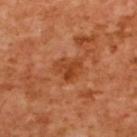Captured during whole-body skin photography for melanoma surveillance; the lesion was not biopsied. From the upper back. An algorithmic analysis of the crop reported a lesion area of about 7 mm², an outline eccentricity of about 0.65 (0 = round, 1 = elongated), and a shape-asymmetry score of about 0.35 (0 = symmetric). The software also gave border irregularity of about 3.5 on a 0–10 scale, a within-lesion color-variation index near 4.5/10, and peripheral color asymmetry of about 1.5. The analysis additionally found a nevus-likeness score of about 25/100 and lesion-presence confidence of about 100/100. The patient is a female in their mid-50s. The recorded lesion diameter is about 3.5 mm. This is a cross-polarized tile. A 15 mm close-up tile from a total-body photography series done for melanoma screening.